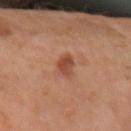workup=total-body-photography surveillance lesion; no biopsy | subject=male, aged around 60 | site=the left forearm | image=total-body-photography crop, ~15 mm field of view | lesion size=about 3.5 mm.